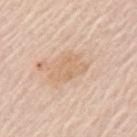No biopsy was performed on this lesion — it was imaged during a full skin examination and was not determined to be concerning.
The lesion is located on the left upper arm.
A roughly 15 mm field-of-view crop from a total-body skin photograph.
Approximately 4.5 mm at its widest.
A male subject aged approximately 65.
This is a white-light tile.